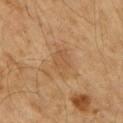biopsy status = total-body-photography surveillance lesion; no biopsy
subject = male, about 60 years old
automated lesion analysis = an area of roughly 6.5 mm² and two-axis asymmetry of about 0.25; a lesion color around L≈48 a*≈18 b*≈33 in CIELAB and a normalized lesion–skin contrast near 4.5; a nevus-likeness score of about 10/100
tile lighting = cross-polarized
location = the right upper arm
imaging modality = ~15 mm crop, total-body skin-cancer survey